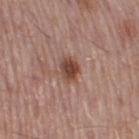Captured during whole-body skin photography for melanoma surveillance; the lesion was not biopsied.
About 3 mm across.
A 15 mm close-up extracted from a 3D total-body photography capture.
Located on the right thigh.
A male patient aged approximately 55.